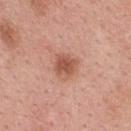follow-up = catalogued during a skin exam; not biopsied | subject = female, in their 40s | lighting = white-light | diameter = about 3 mm | imaging modality = ~15 mm tile from a whole-body skin photo | body site = the back.